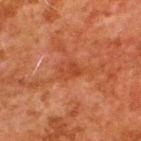Part of a total-body skin-imaging series; this lesion was reviewed on a skin check and was not flagged for biopsy. Approximately 2.5 mm at its widest. A lesion tile, about 15 mm wide, cut from a 3D total-body photograph. Imaged with cross-polarized lighting. A male patient, aged 78–82. The lesion is on the back.